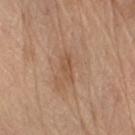A male patient in their 70s.
This image is a 15 mm lesion crop taken from a total-body photograph.
From the left upper arm.